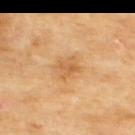Recorded during total-body skin imaging; not selected for excision or biopsy.
On the upper back.
A male patient aged 68 to 72.
This image is a 15 mm lesion crop taken from a total-body photograph.
Approximately 2.5 mm at its widest.